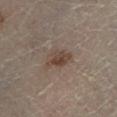Case summary:
– follow-up — imaged on a skin check; not biopsied
– subject — female, roughly 60 years of age
– image-analysis metrics — a border-irregularity index near 2.5/10, internal color variation of about 4 on a 0–10 scale, and peripheral color asymmetry of about 1.5; an automated nevus-likeness rating near 70 out of 100 and lesion-presence confidence of about 100/100
– tile lighting — cross-polarized illumination
– anatomic site — the right lower leg
– diameter — about 3.5 mm
– acquisition — ~15 mm tile from a whole-body skin photo